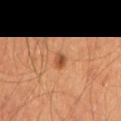- workup — total-body-photography surveillance lesion; no biopsy
- subject — male, in their mid-60s
- image-analysis metrics — a border-irregularity rating of about 2/10 and radial color variation of about 1
- image — ~15 mm tile from a whole-body skin photo
- illumination — cross-polarized illumination
- anatomic site — the right thigh